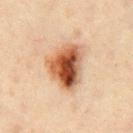follow-up: no biopsy performed (imaged during a skin exam); TBP lesion metrics: a nevus-likeness score of about 100/100; lighting: cross-polarized; patient: male, aged around 40; lesion diameter: ≈5.5 mm; image: ~15 mm crop, total-body skin-cancer survey; body site: the chest.